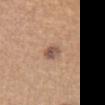notes = total-body-photography surveillance lesion; no biopsy
automated lesion analysis = a shape eccentricity near 0.7; a lesion color around L≈53 a*≈18 b*≈27 in CIELAB, about 12 CIELAB-L* units darker than the surrounding skin, and a normalized lesion–skin contrast near 8.5; a lesion-detection confidence of about 100/100
lighting = white-light illumination
image = total-body-photography crop, ~15 mm field of view
lesion size = ~2.5 mm (longest diameter)
patient = female, aged approximately 25
body site = the left forearm